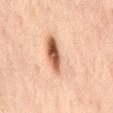Imaged during a routine full-body skin examination; the lesion was not biopsied and no histopathology is available. A region of skin cropped from a whole-body photographic capture, roughly 15 mm wide. This is a cross-polarized tile. Located on the mid back. A female subject aged 53 to 57.